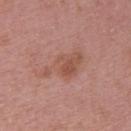<lesion>
<biopsy_status>not biopsied; imaged during a skin examination</biopsy_status>
<lighting>white-light</lighting>
<patient>
  <sex>female</sex>
  <age_approx>45</age_approx>
</patient>
<image>
  <source>total-body photography crop</source>
  <field_of_view_mm>15</field_of_view_mm>
</image>
<site>arm</site>
</lesion>